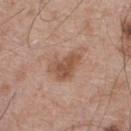| feature | finding |
|---|---|
| biopsy status | total-body-photography surveillance lesion; no biopsy |
| automated metrics | a lesion area of about 10 mm²; a mean CIELAB color near L≈53 a*≈20 b*≈30, about 10 CIELAB-L* units darker than the surrounding skin, and a lesion-to-skin contrast of about 7.5 (normalized; higher = more distinct); a border-irregularity rating of about 4.5/10 and a peripheral color-asymmetry measure near 1 |
| size | ≈4.5 mm |
| tile lighting | white-light |
| body site | the upper back |
| imaging modality | ~15 mm tile from a whole-body skin photo |
| patient | male, about 65 years old |